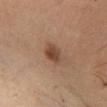  biopsy_status: not biopsied; imaged during a skin examination
  image:
    source: total-body photography crop
    field_of_view_mm: 15
  patient:
    sex: male
    age_approx: 45
  site: leg